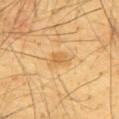Recorded during total-body skin imaging; not selected for excision or biopsy.
The tile uses cross-polarized illumination.
A lesion tile, about 15 mm wide, cut from a 3D total-body photograph.
A male patient, aged around 65.
The lesion is on the upper back.
Longest diameter approximately 3.5 mm.
The lesion-visualizer software estimated a lesion color around L≈67 a*≈19 b*≈47 in CIELAB and a lesion-to-skin contrast of about 5.5 (normalized; higher = more distinct). And it measured a nevus-likeness score of about 0/100 and a detector confidence of about 100 out of 100 that the crop contains a lesion.